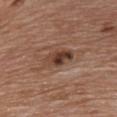Captured during whole-body skin photography for melanoma surveillance; the lesion was not biopsied.
Cropped from a whole-body photographic skin survey; the tile spans about 15 mm.
This is a white-light tile.
Located on the front of the torso.
A male subject aged 78 to 82.